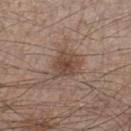<case>
<biopsy_status>not biopsied; imaged during a skin examination</biopsy_status>
<patient>
  <sex>male</sex>
  <age_approx>45</age_approx>
</patient>
<lighting>white-light</lighting>
<automated_metrics>
  <border_irregularity_0_10>3.0</border_irregularity_0_10>
  <color_variation_0_10>3.0</color_variation_0_10>
  <nevus_likeness_0_100>65</nevus_likeness_0_100>
  <lesion_detection_confidence_0_100>100</lesion_detection_confidence_0_100>
</automated_metrics>
<lesion_size>
  <long_diameter_mm_approx>3.5</long_diameter_mm_approx>
</lesion_size>
<image>
  <source>total-body photography crop</source>
  <field_of_view_mm>15</field_of_view_mm>
</image>
<site>right lower leg</site>
</case>